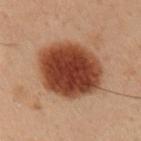Case summary:
– workup: no biopsy performed (imaged during a skin exam)
– anatomic site: the right upper arm
– image: ~15 mm crop, total-body skin-cancer survey
– tile lighting: cross-polarized illumination
– lesion diameter: about 6.5 mm
– patient: male, approximately 55 years of age
– automated metrics: an area of roughly 33 mm² and an outline eccentricity of about 0.25 (0 = round, 1 = elongated)